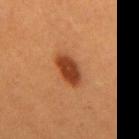<case>
<biopsy_status>not biopsied; imaged during a skin examination</biopsy_status>
<lighting>cross-polarized</lighting>
<patient>
  <sex>male</sex>
  <age_approx>60</age_approx>
</patient>
<lesion_size>
  <long_diameter_mm_approx>4.0</long_diameter_mm_approx>
</lesion_size>
<automated_metrics>
  <cielab_L>39</cielab_L>
  <cielab_a>27</cielab_a>
  <cielab_b>36</cielab_b>
  <vs_skin_darker_L>14.0</vs_skin_darker_L>
  <vs_skin_contrast_norm>11.0</vs_skin_contrast_norm>
  <lesion_detection_confidence_0_100>100</lesion_detection_confidence_0_100>
</automated_metrics>
<image>
  <source>total-body photography crop</source>
  <field_of_view_mm>15</field_of_view_mm>
</image>
<site>back</site>
</case>